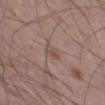Q: Was this lesion biopsied?
A: total-body-photography surveillance lesion; no biopsy